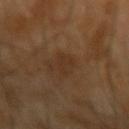{
  "biopsy_status": "not biopsied; imaged during a skin examination"
}